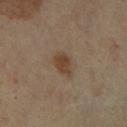notes: no biopsy performed (imaged during a skin exam) | diameter: about 3 mm | body site: the right lower leg | image source: total-body-photography crop, ~15 mm field of view | patient: aged 58–62 | lighting: cross-polarized illumination | automated metrics: an area of roughly 5 mm² and a shape eccentricity near 0.75; about 9 CIELAB-L* units darker than the surrounding skin and a normalized border contrast of about 8; a color-variation rating of about 2/10 and a peripheral color-asymmetry measure near 0.5; a classifier nevus-likeness of about 85/100 and a detector confidence of about 100 out of 100 that the crop contains a lesion.